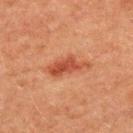Case summary:
- follow-up · imaged on a skin check; not biopsied
- patient · male, approximately 50 years of age
- acquisition · ~15 mm crop, total-body skin-cancer survey
- lesion size · about 5 mm
- anatomic site · the upper back
- automated lesion analysis · an eccentricity of roughly 0.9 and two-axis asymmetry of about 0.4; a border-irregularity index near 5/10, a color-variation rating of about 2/10, and radial color variation of about 0.5
- illumination · cross-polarized illumination